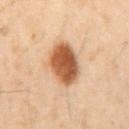The lesion was tiled from a total-body skin photograph and was not biopsied. Automated tile analysis of the lesion measured an area of roughly 14 mm², an eccentricity of roughly 0.65, and two-axis asymmetry of about 0.15. The analysis additionally found border irregularity of about 1.5 on a 0–10 scale and a peripheral color-asymmetry measure near 1. A close-up tile cropped from a whole-body skin photograph, about 15 mm across. Located on the front of the torso. A male subject in their 50s.